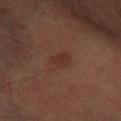Assessment: The lesion was photographed on a routine skin check and not biopsied; there is no pathology result. Acquisition and patient details: The lesion-visualizer software estimated an area of roughly 4 mm², a shape eccentricity near 0.75, and a shape-asymmetry score of about 0.25 (0 = symmetric). The analysis additionally found a mean CIELAB color near L≈26 a*≈17 b*≈22, about 5 CIELAB-L* units darker than the surrounding skin, and a normalized border contrast of about 6. And it measured a classifier nevus-likeness of about 20/100. The tile uses cross-polarized illumination. The subject is a male about 85 years old or older. On the left forearm. A roughly 15 mm field-of-view crop from a total-body skin photograph. Measured at roughly 2.5 mm in maximum diameter.